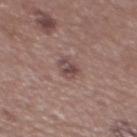{
  "biopsy_status": "not biopsied; imaged during a skin examination",
  "site": "chest",
  "lesion_size": {
    "long_diameter_mm_approx": 2.5
  },
  "image": {
    "source": "total-body photography crop",
    "field_of_view_mm": 15
  },
  "patient": {
    "sex": "male",
    "age_approx": 55
  }
}